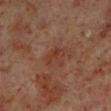Recorded during total-body skin imaging; not selected for excision or biopsy. Located on the left lower leg. A 15 mm close-up tile from a total-body photography series done for melanoma screening. This is a cross-polarized tile. The total-body-photography lesion software estimated an area of roughly 3.5 mm² and a shape eccentricity near 0.85. The analysis additionally found a mean CIELAB color near L≈34 a*≈22 b*≈26, a lesion–skin lightness drop of about 6, and a normalized lesion–skin contrast near 5.5. The software also gave border irregularity of about 4.5 on a 0–10 scale and a within-lesion color-variation index near 2/10. The subject is a male aged 58 to 62.